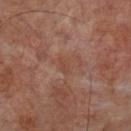Notes:
* workup · no biopsy performed (imaged during a skin exam)
* lesion size · ~2.5 mm (longest diameter)
* lighting · cross-polarized
* imaging modality · ~15 mm tile from a whole-body skin photo
* patient · male, in their 70s
* automated metrics · a detector confidence of about 95 out of 100 that the crop contains a lesion
* location · the leg